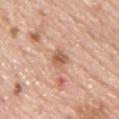Clinical impression:
Recorded during total-body skin imaging; not selected for excision or biopsy.
Background:
Automated image analysis of the tile measured a within-lesion color-variation index near 3/10 and radial color variation of about 1. The lesion's longest dimension is about 2.5 mm. This image is a 15 mm lesion crop taken from a total-body photograph. The tile uses white-light illumination. The lesion is on the chest. A male patient, aged 43 to 47.